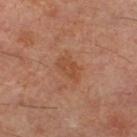Recorded during total-body skin imaging; not selected for excision or biopsy.
Approximately 3.5 mm at its widest.
On the left lower leg.
A male patient, aged approximately 60.
A region of skin cropped from a whole-body photographic capture, roughly 15 mm wide.
The tile uses cross-polarized illumination.
Automated tile analysis of the lesion measured an eccentricity of roughly 0.85 and a symmetry-axis asymmetry near 0.3. The software also gave a classifier nevus-likeness of about 15/100 and a lesion-detection confidence of about 100/100.